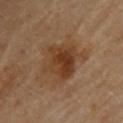The lesion's longest dimension is about 5.5 mm. Located on the upper back. The tile uses cross-polarized illumination. A region of skin cropped from a whole-body photographic capture, roughly 15 mm wide. A female subject, aged 68 to 72.